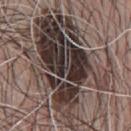Notes:
* patient: male, aged approximately 60
* lighting: white-light illumination
* anatomic site: the chest
* image-analysis metrics: an area of roughly 65 mm², an eccentricity of roughly 0.85, and a symmetry-axis asymmetry near 0.4; a classifier nevus-likeness of about 15/100
* image source: 15 mm crop, total-body photography
* diameter: ~14.5 mm (longest diameter)
* histopathology: a dysplastic (Clark) nevus